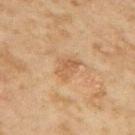Q: What kind of image is this?
A: ~15 mm tile from a whole-body skin photo
Q: Who is the patient?
A: female, aged 58 to 62
Q: What is the anatomic site?
A: the upper back
Q: Lesion size?
A: about 3 mm